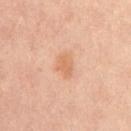Notes:
– follow-up — no biopsy performed (imaged during a skin exam)
– image — ~15 mm crop, total-body skin-cancer survey
– lesion diameter — ~3 mm (longest diameter)
– subject — female, aged around 50
– site — the mid back
– illumination — cross-polarized illumination